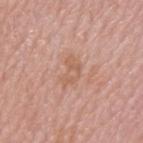follow-up = no biopsy performed (imaged during a skin exam); subject = male, approximately 50 years of age; lighting = white-light illumination; anatomic site = the upper back; imaging modality = 15 mm crop, total-body photography; TBP lesion metrics = border irregularity of about 4.5 on a 0–10 scale, internal color variation of about 2.5 on a 0–10 scale, and peripheral color asymmetry of about 1.